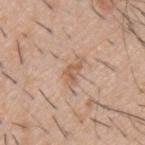Assessment:
The lesion was photographed on a routine skin check and not biopsied; there is no pathology result.